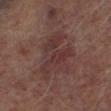| field | value |
|---|---|
| notes | catalogued during a skin exam; not biopsied |
| tile lighting | cross-polarized illumination |
| site | the left lower leg |
| subject | male, aged 63 to 67 |
| acquisition | 15 mm crop, total-body photography |